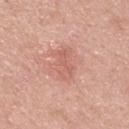Q: Was this lesion biopsied?
A: no biopsy performed (imaged during a skin exam)
Q: What kind of image is this?
A: ~15 mm crop, total-body skin-cancer survey
Q: Lesion location?
A: the back
Q: What are the patient's age and sex?
A: male, roughly 45 years of age